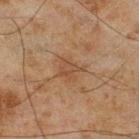Clinical impression: No biopsy was performed on this lesion — it was imaged during a full skin examination and was not determined to be concerning. Image and clinical context: On the leg. A 15 mm crop from a total-body photograph taken for skin-cancer surveillance. A male subject aged around 45.